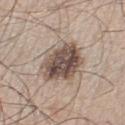Clinical impression:
No biopsy was performed on this lesion — it was imaged during a full skin examination and was not determined to be concerning.
Acquisition and patient details:
The lesion is located on the left lower leg. Measured at roughly 6 mm in maximum diameter. The lesion-visualizer software estimated an automated nevus-likeness rating near 30 out of 100 and lesion-presence confidence of about 100/100. Captured under white-light illumination. A male patient in their mid-40s. Cropped from a whole-body photographic skin survey; the tile spans about 15 mm.